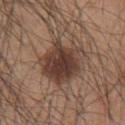biopsy_status: not biopsied; imaged during a skin examination
site: left upper arm
lesion_size:
  long_diameter_mm_approx: 6.5
image:
  source: total-body photography crop
  field_of_view_mm: 15
patient:
  sex: male
  age_approx: 45